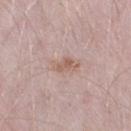• follow-up · total-body-photography surveillance lesion; no biopsy
• patient · male, roughly 50 years of age
• acquisition · total-body-photography crop, ~15 mm field of view
• body site · the right thigh
• size · ≈3.5 mm